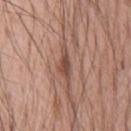Q: Was this lesion biopsied?
A: no biopsy performed (imaged during a skin exam)
Q: What lighting was used for the tile?
A: white-light
Q: What kind of image is this?
A: ~15 mm tile from a whole-body skin photo
Q: What is the lesion's diameter?
A: ~4.5 mm (longest diameter)
Q: Lesion location?
A: the chest
Q: Who is the patient?
A: male, aged approximately 55